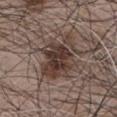Q: Was a biopsy performed?
A: no biopsy performed (imaged during a skin exam)
Q: What lighting was used for the tile?
A: white-light
Q: Patient demographics?
A: male, about 65 years old
Q: How was this image acquired?
A: ~15 mm crop, total-body skin-cancer survey
Q: Lesion location?
A: the chest